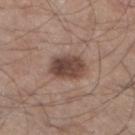<lesion>
  <biopsy_status>not biopsied; imaged during a skin examination</biopsy_status>
  <lighting>white-light</lighting>
  <patient>
    <sex>male</sex>
    <age_approx>45</age_approx>
  </patient>
  <site>left lower leg</site>
  <image>
    <source>total-body photography crop</source>
    <field_of_view_mm>15</field_of_view_mm>
  </image>
</lesion>